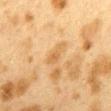Q: Is there a histopathology result?
A: catalogued during a skin exam; not biopsied
Q: How was this image acquired?
A: 15 mm crop, total-body photography
Q: Who is the patient?
A: female, aged approximately 40
Q: Lesion location?
A: the mid back